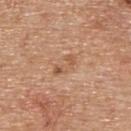The lesion was photographed on a routine skin check and not biopsied; there is no pathology result.
This is a white-light tile.
From the upper back.
A 15 mm crop from a total-body photograph taken for skin-cancer surveillance.
The lesion-visualizer software estimated a lesion color around L≈56 a*≈21 b*≈33 in CIELAB, a lesion–skin lightness drop of about 8, and a normalized lesion–skin contrast near 5.5. The software also gave a border-irregularity rating of about 4.5/10 and radial color variation of about 2. And it measured lesion-presence confidence of about 100/100.
The recorded lesion diameter is about 3.5 mm.
A male subject, aged around 65.